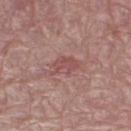biopsy_status: not biopsied; imaged during a skin examination
patient:
  sex: female
  age_approx: 65
site: right thigh
lesion_size:
  long_diameter_mm_approx: 2.5
image:
  source: total-body photography crop
  field_of_view_mm: 15
automated_metrics:
  area_mm2_approx: 4.5
  eccentricity: 0.65
  shape_asymmetry: 0.25